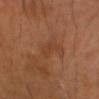notes = total-body-photography surveillance lesion; no biopsy | lesion diameter = ~3 mm (longest diameter) | tile lighting = cross-polarized | patient = female, roughly 50 years of age | TBP lesion metrics = a shape eccentricity near 0.9 and a symmetry-axis asymmetry near 0.3; roughly 5 lightness units darker than nearby skin and a normalized border contrast of about 4.5; a border-irregularity index near 4/10 and internal color variation of about 0.5 on a 0–10 scale | acquisition = total-body-photography crop, ~15 mm field of view.